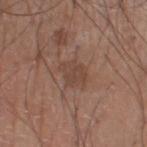Imaged during a routine full-body skin examination; the lesion was not biopsied and no histopathology is available. Approximately 3 mm at its widest. The subject is a male roughly 60 years of age. Cropped from a whole-body photographic skin survey; the tile spans about 15 mm. The lesion is located on the right lower leg.